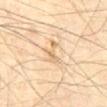{
  "biopsy_status": "not biopsied; imaged during a skin examination",
  "image": {
    "source": "total-body photography crop",
    "field_of_view_mm": 15
  },
  "site": "abdomen",
  "lighting": "cross-polarized",
  "patient": {
    "sex": "male",
    "age_approx": 70
  },
  "lesion_size": {
    "long_diameter_mm_approx": 3.0
  },
  "automated_metrics": {
    "cielab_L": 71,
    "cielab_a": 16,
    "cielab_b": 38,
    "vs_skin_darker_L": 9.0,
    "vs_skin_contrast_norm": 5.5,
    "border_irregularity_0_10": 7.0,
    "color_variation_0_10": 1.0,
    "peripheral_color_asymmetry": 0.0
  }
}